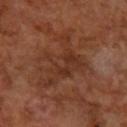Captured during whole-body skin photography for melanoma surveillance; the lesion was not biopsied. On the right forearm. This is a cross-polarized tile. A 15 mm close-up extracted from a 3D total-body photography capture. A female subject about 65 years old.